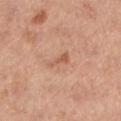follow-up: catalogued during a skin exam; not biopsied
site: the left lower leg
tile lighting: white-light
subject: female, aged 38 to 42
diameter: ≈2.5 mm
imaging modality: 15 mm crop, total-body photography